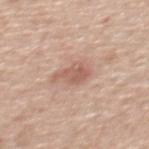| feature | finding |
|---|---|
| notes | imaged on a skin check; not biopsied |
| image-analysis metrics | an average lesion color of about L≈59 a*≈21 b*≈28 (CIELAB), a lesion–skin lightness drop of about 10, and a normalized lesion–skin contrast near 6.5; a border-irregularity rating of about 4/10, internal color variation of about 2.5 on a 0–10 scale, and radial color variation of about 1; a nevus-likeness score of about 5/100 and a lesion-detection confidence of about 100/100 |
| body site | the upper back |
| image source | 15 mm crop, total-body photography |
| lesion size | ≈4 mm |
| patient | male, aged approximately 60 |
| lighting | white-light |